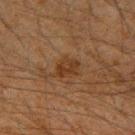The lesion was photographed on a routine skin check and not biopsied; there is no pathology result. The patient is a male in their mid- to late 30s. Captured under cross-polarized illumination. From the right upper arm. The lesion-visualizer software estimated an average lesion color of about L≈27 a*≈16 b*≈26 (CIELAB), about 6 CIELAB-L* units darker than the surrounding skin, and a lesion-to-skin contrast of about 7 (normalized; higher = more distinct). The software also gave a lesion-detection confidence of about 100/100. The recorded lesion diameter is about 3 mm. A 15 mm close-up tile from a total-body photography series done for melanoma screening.